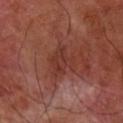Q: Is there a histopathology result?
A: total-body-photography surveillance lesion; no biopsy